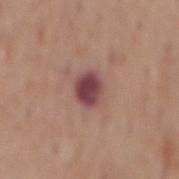Recorded during total-body skin imaging; not selected for excision or biopsy. Cropped from a total-body skin-imaging series; the visible field is about 15 mm. A male patient, aged around 70. The lesion is on the mid back. Measured at roughly 3.5 mm in maximum diameter. Automated image analysis of the tile measured roughly 14 lightness units darker than nearby skin and a lesion-to-skin contrast of about 11.5 (normalized; higher = more distinct). It also reported a border-irregularity index near 1.5/10 and a peripheral color-asymmetry measure near 1.